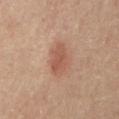notes — imaged on a skin check; not biopsied | acquisition — 15 mm crop, total-body photography | subject — male, roughly 85 years of age or older | tile lighting — cross-polarized illumination | lesion size — ≈4 mm | anatomic site — the mid back.